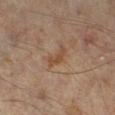follow-up — imaged on a skin check; not biopsied | site — the right lower leg | imaging modality — ~15 mm tile from a whole-body skin photo | subject — male, roughly 65 years of age.